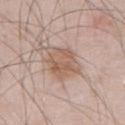{
  "biopsy_status": "not biopsied; imaged during a skin examination",
  "lighting": "white-light",
  "image": {
    "source": "total-body photography crop",
    "field_of_view_mm": 15
  },
  "site": "abdomen",
  "patient": {
    "sex": "male",
    "age_approx": 55
  }
}